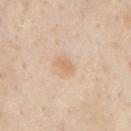workup = no biopsy performed (imaged during a skin exam)
diameter = about 2.5 mm
automated metrics = an average lesion color of about L≈68 a*≈16 b*≈35 (CIELAB) and a lesion–skin lightness drop of about 7; a border-irregularity rating of about 2/10, a color-variation rating of about 1.5/10, and peripheral color asymmetry of about 0.5
acquisition = total-body-photography crop, ~15 mm field of view
patient = male, about 55 years old
site = the chest